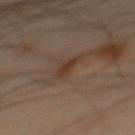This image is a 15 mm lesion crop taken from a total-body photograph.
The subject is a male aged 48 to 52.
The lesion is on the back.